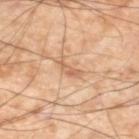The patient is a male aged around 55. Measured at roughly 3.5 mm in maximum diameter. This is a cross-polarized tile. Cropped from a total-body skin-imaging series; the visible field is about 15 mm. Located on the left lower leg.